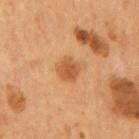| key | value |
|---|---|
| tile lighting | cross-polarized |
| anatomic site | the mid back |
| lesion size | ~3 mm (longest diameter) |
| acquisition | total-body-photography crop, ~15 mm field of view |
| patient | male, aged 53 to 57 |